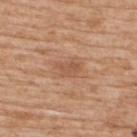Part of a total-body skin-imaging series; this lesion was reviewed on a skin check and was not flagged for biopsy.
A close-up tile cropped from a whole-body skin photograph, about 15 mm across.
Approximately 2.5 mm at its widest.
Located on the upper back.
Automated tile analysis of the lesion measured a footprint of about 4 mm² and a shape-asymmetry score of about 0.4 (0 = symmetric). It also reported an average lesion color of about L≈54 a*≈22 b*≈33 (CIELAB) and a lesion–skin lightness drop of about 8. It also reported a border-irregularity rating of about 3.5/10 and internal color variation of about 1 on a 0–10 scale. The software also gave a nevus-likeness score of about 0/100 and lesion-presence confidence of about 100/100.
A male subject aged 58 to 62.